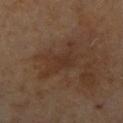The lesion was tiled from a total-body skin photograph and was not biopsied.
Longest diameter approximately 5.5 mm.
A 15 mm close-up tile from a total-body photography series done for melanoma screening.
From the left leg.
A female patient, in their mid- to late 60s.
Captured under cross-polarized illumination.